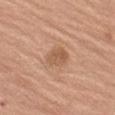Part of a total-body skin-imaging series; this lesion was reviewed on a skin check and was not flagged for biopsy. The lesion is on the leg. The tile uses white-light illumination. A female subject, roughly 70 years of age. A close-up tile cropped from a whole-body skin photograph, about 15 mm across.